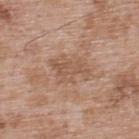Captured during whole-body skin photography for melanoma surveillance; the lesion was not biopsied. The patient is a male aged approximately 50. Measured at roughly 5 mm in maximum diameter. On the upper back. A 15 mm crop from a total-body photograph taken for skin-cancer surveillance. The tile uses white-light illumination.